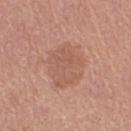Assessment: The lesion was photographed on a routine skin check and not biopsied; there is no pathology result. Clinical summary: A lesion tile, about 15 mm wide, cut from a 3D total-body photograph. The recorded lesion diameter is about 5 mm. The total-body-photography lesion software estimated a lesion color around L≈57 a*≈23 b*≈28 in CIELAB, roughly 6 lightness units darker than nearby skin, and a normalized border contrast of about 4.5. It also reported border irregularity of about 2 on a 0–10 scale, a color-variation rating of about 2.5/10, and peripheral color asymmetry of about 1. The software also gave a nevus-likeness score of about 0/100 and a detector confidence of about 100 out of 100 that the crop contains a lesion. The tile uses white-light illumination. On the right thigh. A female patient aged 53–57.